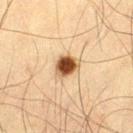* biopsy status · total-body-photography surveillance lesion; no biopsy
* automated lesion analysis · a nevus-likeness score of about 100/100 and lesion-presence confidence of about 100/100
* site · the left thigh
* size · about 3 mm
* subject · male, in their 50s
* image source · ~15 mm crop, total-body skin-cancer survey